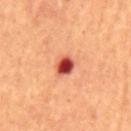lesion diameter: about 3 mm | automated metrics: a lesion area of about 5 mm²; a lesion color around L≈47 a*≈35 b*≈32 in CIELAB, about 18 CIELAB-L* units darker than the surrounding skin, and a lesion-to-skin contrast of about 12.5 (normalized; higher = more distinct); a border-irregularity index near 1.5/10 and internal color variation of about 7 on a 0–10 scale; a nevus-likeness score of about 0/100 and a detector confidence of about 100 out of 100 that the crop contains a lesion | image source: total-body-photography crop, ~15 mm field of view | lighting: cross-polarized | patient: male, aged approximately 65 | location: the abdomen.